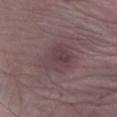Part of a total-body skin-imaging series; this lesion was reviewed on a skin check and was not flagged for biopsy. From the right lower leg. The tile uses white-light illumination. An algorithmic analysis of the crop reported an average lesion color of about L≈40 a*≈18 b*≈12 (CIELAB), about 7 CIELAB-L* units darker than the surrounding skin, and a lesion-to-skin contrast of about 6 (normalized; higher = more distinct). The software also gave border irregularity of about 2.5 on a 0–10 scale, a color-variation rating of about 3.5/10, and peripheral color asymmetry of about 1. The analysis additionally found an automated nevus-likeness rating near 0 out of 100 and a detector confidence of about 100 out of 100 that the crop contains a lesion. A male patient roughly 65 years of age. A lesion tile, about 15 mm wide, cut from a 3D total-body photograph. Approximately 5 mm at its widest.